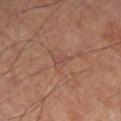The lesion was tiled from a total-body skin photograph and was not biopsied. Approximately 2.5 mm at its widest. The subject is a male aged approximately 60. A region of skin cropped from a whole-body photographic capture, roughly 15 mm wide. The lesion is on the right lower leg. The tile uses cross-polarized illumination.